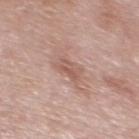This lesion was catalogued during total-body skin photography and was not selected for biopsy. The lesion's longest dimension is about 3 mm. The lesion-visualizer software estimated a footprint of about 5 mm² and an eccentricity of roughly 0.7. It also reported an average lesion color of about L≈57 a*≈21 b*≈25 (CIELAB), a lesion–skin lightness drop of about 8, and a lesion-to-skin contrast of about 5.5 (normalized; higher = more distinct). Located on the front of the torso. A male subject about 55 years old. A 15 mm close-up extracted from a 3D total-body photography capture. Imaged with white-light lighting.